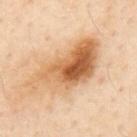No biopsy was performed on this lesion — it was imaged during a full skin examination and was not determined to be concerning. The lesion-visualizer software estimated a lesion area of about 30 mm², an outline eccentricity of about 0.9 (0 = round, 1 = elongated), and a symmetry-axis asymmetry near 0.4. The analysis additionally found a detector confidence of about 100 out of 100 that the crop contains a lesion. Approximately 10 mm at its widest. The lesion is on the mid back. A 15 mm close-up extracted from a 3D total-body photography capture. The tile uses cross-polarized illumination. A male patient aged approximately 55.